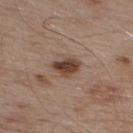Q: Was this lesion biopsied?
A: imaged on a skin check; not biopsied
Q: What is the lesion's diameter?
A: about 3.5 mm
Q: Who is the patient?
A: male, aged 53–57
Q: Illumination type?
A: white-light
Q: Where on the body is the lesion?
A: the upper back
Q: What is the imaging modality?
A: ~15 mm tile from a whole-body skin photo